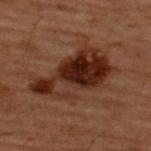Acquisition and patient details: Located on the upper back. Captured under cross-polarized illumination. An algorithmic analysis of the crop reported a footprint of about 30 mm², a shape eccentricity near 0.85, and two-axis asymmetry of about 0.3. And it measured an average lesion color of about L≈20 a*≈17 b*≈21 (CIELAB) and a normalized border contrast of about 11. The software also gave a classifier nevus-likeness of about 15/100 and lesion-presence confidence of about 100/100. A male subject, aged approximately 60. Approximately 8.5 mm at its widest. A 15 mm close-up extracted from a 3D total-body photography capture.